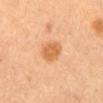• notes: catalogued during a skin exam; not biopsied
• imaging modality: ~15 mm tile from a whole-body skin photo
• size: ~3 mm (longest diameter)
• location: the mid back
• lighting: cross-polarized
• patient: female, aged around 60